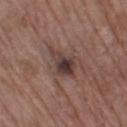Assessment:
This lesion was catalogued during total-body skin photography and was not selected for biopsy.